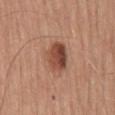{"biopsy_status": "not biopsied; imaged during a skin examination", "image": {"source": "total-body photography crop", "field_of_view_mm": 15}, "automated_metrics": {"border_irregularity_0_10": 1.5, "color_variation_0_10": 7.0, "nevus_likeness_0_100": 95, "lesion_detection_confidence_0_100": 100}, "patient": {"sex": "male", "age_approx": 55}, "lesion_size": {"long_diameter_mm_approx": 4.0}, "site": "mid back", "lighting": "white-light"}